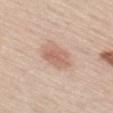Findings:
* image: total-body-photography crop, ~15 mm field of view
* location: the leg
* lesion size: ~4.5 mm (longest diameter)
* patient: male, aged 58 to 62